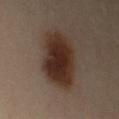Context: This is a cross-polarized tile. A female patient, roughly 40 years of age. The lesion-visualizer software estimated a border-irregularity rating of about 2/10, internal color variation of about 4.5 on a 0–10 scale, and radial color variation of about 1.5. Located on the arm. Measured at roughly 7 mm in maximum diameter. A roughly 15 mm field-of-view crop from a total-body skin photograph.